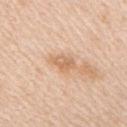follow-up = catalogued during a skin exam; not biopsied | automated metrics = a border-irregularity index near 2.5/10, a within-lesion color-variation index near 3/10, and radial color variation of about 1 | subject = female, approximately 45 years of age | size = ≈3 mm | image = 15 mm crop, total-body photography | location = the right upper arm.